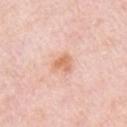No biopsy was performed on this lesion — it was imaged during a full skin examination and was not determined to be concerning. A female patient about 65 years old. Cropped from a total-body skin-imaging series; the visible field is about 15 mm. From the right upper arm. Longest diameter approximately 2.5 mm.